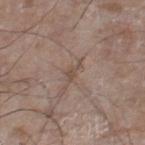follow-up: no biopsy performed (imaged during a skin exam) | anatomic site: the right lower leg | image: 15 mm crop, total-body photography | size: ~3 mm (longest diameter) | subject: male, roughly 60 years of age | lighting: white-light illumination | automated metrics: an average lesion color of about L≈49 a*≈14 b*≈24 (CIELAB), roughly 7 lightness units darker than nearby skin, and a normalized border contrast of about 5.5; an automated nevus-likeness rating near 0 out of 100.